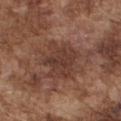{
  "lighting": "white-light",
  "lesion_size": {
    "long_diameter_mm_approx": 6.5
  },
  "image": {
    "source": "total-body photography crop",
    "field_of_view_mm": 15
  },
  "patient": {
    "sex": "male",
    "age_approx": 75
  },
  "site": "chest"
}